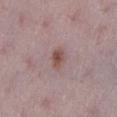Context:
On the left lower leg. The subject is a female about 50 years old. This is a white-light tile. A 15 mm crop from a total-body photograph taken for skin-cancer surveillance.